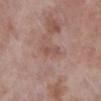{"biopsy_status": "not biopsied; imaged during a skin examination", "lesion_size": {"long_diameter_mm_approx": 3.0}, "patient": {"sex": "female", "age_approx": 70}, "lighting": "white-light", "image": {"source": "total-body photography crop", "field_of_view_mm": 15}, "site": "right lower leg"}